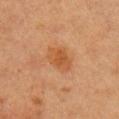Impression:
The lesion was tiled from a total-body skin photograph and was not biopsied.
Clinical summary:
The tile uses cross-polarized illumination. Approximately 3.5 mm at its widest. A female patient aged around 40. A lesion tile, about 15 mm wide, cut from a 3D total-body photograph. The lesion is on the arm. Automated tile analysis of the lesion measured an area of roughly 7.5 mm² and a shape eccentricity near 0.7. And it measured an average lesion color of about L≈46 a*≈22 b*≈35 (CIELAB).